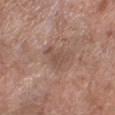• workup · imaged on a skin check; not biopsied
• image · 15 mm crop, total-body photography
• patient · female, aged 58–62
• diameter · ~3.5 mm (longest diameter)
• site · the left forearm